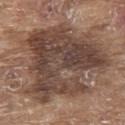– workup: total-body-photography surveillance lesion; no biopsy
– anatomic site: the upper back
– acquisition: total-body-photography crop, ~15 mm field of view
– patient: male, about 80 years old
– lesion size: about 11.5 mm